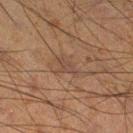Clinical impression: Imaged during a routine full-body skin examination; the lesion was not biopsied and no histopathology is available. Acquisition and patient details: A roughly 15 mm field-of-view crop from a total-body skin photograph. This is a cross-polarized tile. The patient is a male approximately 35 years of age. Located on the leg.